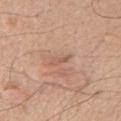| field | value |
|---|---|
| lesion diameter | ~2.5 mm (longest diameter) |
| image source | ~15 mm tile from a whole-body skin photo |
| subject | male, aged 73 to 77 |
| body site | the front of the torso |
| image-analysis metrics | a mean CIELAB color near L≈57 a*≈21 b*≈28, about 8 CIELAB-L* units darker than the surrounding skin, and a lesion-to-skin contrast of about 5 (normalized; higher = more distinct); a border-irregularity index near 4.5/10, internal color variation of about 0 on a 0–10 scale, and radial color variation of about 0; a classifier nevus-likeness of about 0/100 and a detector confidence of about 80 out of 100 that the crop contains a lesion |